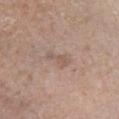Longest diameter approximately 3.5 mm. Located on the leg. Captured under white-light illumination. Cropped from a whole-body photographic skin survey; the tile spans about 15 mm. A male subject, in their mid-70s. The lesion-visualizer software estimated an outline eccentricity of about 0.9 (0 = round, 1 = elongated) and a symmetry-axis asymmetry near 0.45. The analysis additionally found a nevus-likeness score of about 0/100 and lesion-presence confidence of about 100/100.